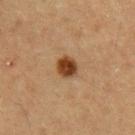Assessment: Captured during whole-body skin photography for melanoma surveillance; the lesion was not biopsied. Background: A close-up tile cropped from a whole-body skin photograph, about 15 mm across. A male patient about 60 years old.